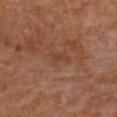Assessment: No biopsy was performed on this lesion — it was imaged during a full skin examination and was not determined to be concerning. Clinical summary: Measured at roughly 2.5 mm in maximum diameter. On the upper back. Automated tile analysis of the lesion measured a lesion area of about 2.5 mm² and a shape eccentricity near 0.9. The analysis additionally found internal color variation of about 0.5 on a 0–10 scale and radial color variation of about 0. The patient is female. A roughly 15 mm field-of-view crop from a total-body skin photograph.